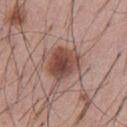patient = male, aged 53–57
body site = the abdomen
image source = 15 mm crop, total-body photography